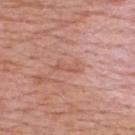The lesion was tiled from a total-body skin photograph and was not biopsied. Imaged with white-light lighting. A close-up tile cropped from a whole-body skin photograph, about 15 mm across. A male subject roughly 65 years of age. Located on the upper back. Automated tile analysis of the lesion measured an outline eccentricity of about 0.9 (0 = round, 1 = elongated) and two-axis asymmetry of about 0.55. The analysis additionally found about 6 CIELAB-L* units darker than the surrounding skin and a normalized border contrast of about 5. It also reported internal color variation of about 0 on a 0–10 scale and a peripheral color-asymmetry measure near 0. Longest diameter approximately 3.5 mm.